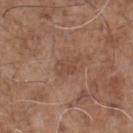image source: ~15 mm crop, total-body skin-cancer survey
subject: male, aged 68–72
body site: the chest
illumination: white-light
size: about 3 mm